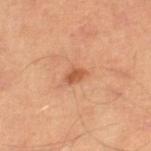Notes:
* notes — total-body-photography surveillance lesion; no biopsy
* image source — total-body-photography crop, ~15 mm field of view
* patient — male, aged approximately 65
* body site — the right lower leg